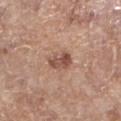Q: Was this lesion biopsied?
A: imaged on a skin check; not biopsied
Q: How was this image acquired?
A: ~15 mm crop, total-body skin-cancer survey
Q: Where on the body is the lesion?
A: the left lower leg
Q: Who is the patient?
A: female, in their mid- to late 70s